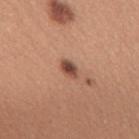Part of a total-body skin-imaging series; this lesion was reviewed on a skin check and was not flagged for biopsy. An algorithmic analysis of the crop reported a lesion area of about 3.5 mm², an outline eccentricity of about 0.8 (0 = round, 1 = elongated), and a symmetry-axis asymmetry near 0.2. The analysis additionally found a lesion-detection confidence of about 100/100. The lesion is located on the back. A female subject approximately 30 years of age. Measured at roughly 2.5 mm in maximum diameter. A 15 mm crop from a total-body photograph taken for skin-cancer surveillance. This is a white-light tile.